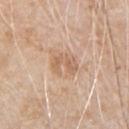No biopsy was performed on this lesion — it was imaged during a full skin examination and was not determined to be concerning. The lesion is located on the chest. The recorded lesion diameter is about 3 mm. The tile uses white-light illumination. A roughly 15 mm field-of-view crop from a total-body skin photograph. A male patient roughly 80 years of age.